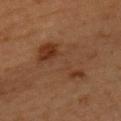{"biopsy_status": "not biopsied; imaged during a skin examination", "image": {"source": "total-body photography crop", "field_of_view_mm": 15}, "lesion_size": {"long_diameter_mm_approx": 7.0}, "patient": {"sex": "male", "age_approx": 60}, "lighting": "cross-polarized", "site": "chest"}